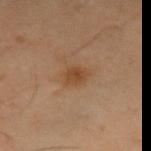biopsy_status: not biopsied; imaged during a skin examination
image:
  source: total-body photography crop
  field_of_view_mm: 15
patient:
  sex: male
  age_approx: 60
lighting: cross-polarized
lesion_size:
  long_diameter_mm_approx: 2.5
site: left thigh
automated_metrics:
  area_mm2_approx: 4.0
  shape_asymmetry: 0.2
  border_irregularity_0_10: 2.0
  color_variation_0_10: 2.0
  peripheral_color_asymmetry: 0.5